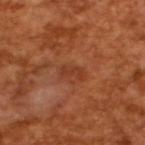The lesion was tiled from a total-body skin photograph and was not biopsied. A male patient about 65 years old. A lesion tile, about 15 mm wide, cut from a 3D total-body photograph. The total-body-photography lesion software estimated a border-irregularity index near 5/10, a color-variation rating of about 0/10, and radial color variation of about 0. The software also gave lesion-presence confidence of about 100/100. About 2.5 mm across.